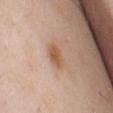Part of a total-body skin-imaging series; this lesion was reviewed on a skin check and was not flagged for biopsy. An algorithmic analysis of the crop reported a border-irregularity index near 2/10, internal color variation of about 3 on a 0–10 scale, and radial color variation of about 1. The analysis additionally found a nevus-likeness score of about 85/100 and a detector confidence of about 100 out of 100 that the crop contains a lesion. Cropped from a total-body skin-imaging series; the visible field is about 15 mm. Longest diameter approximately 3 mm. A male subject aged 53 to 57. The lesion is on the chest. Imaged with white-light lighting.